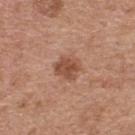The lesion was tiled from a total-body skin photograph and was not biopsied. The lesion's longest dimension is about 3 mm. A female subject aged 38 to 42. On the upper back. Imaged with white-light lighting. The total-body-photography lesion software estimated a footprint of about 7 mm², an outline eccentricity of about 0.3 (0 = round, 1 = elongated), and a symmetry-axis asymmetry near 0.2. And it measured a mean CIELAB color near L≈50 a*≈23 b*≈30, a lesion–skin lightness drop of about 10, and a normalized border contrast of about 7.5. A close-up tile cropped from a whole-body skin photograph, about 15 mm across.